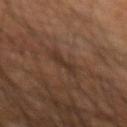Imaged during a routine full-body skin examination; the lesion was not biopsied and no histopathology is available. The lesion is on the arm. This image is a 15 mm lesion crop taken from a total-body photograph. The subject is a male roughly 60 years of age.